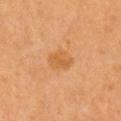Part of a total-body skin-imaging series; this lesion was reviewed on a skin check and was not flagged for biopsy.
A male patient, in their 60s.
Imaged with cross-polarized lighting.
The recorded lesion diameter is about 3 mm.
The lesion is located on the right upper arm.
Cropped from a whole-body photographic skin survey; the tile spans about 15 mm.